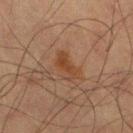{
  "biopsy_status": "not biopsied; imaged during a skin examination",
  "lighting": "cross-polarized",
  "image": {
    "source": "total-body photography crop",
    "field_of_view_mm": 15
  },
  "site": "left thigh",
  "patient": {
    "sex": "male",
    "age_approx": 65
  },
  "lesion_size": {
    "long_diameter_mm_approx": 4.0
  }
}